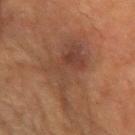Captured during whole-body skin photography for melanoma surveillance; the lesion was not biopsied.
The lesion is on the right forearm.
A female patient, roughly 80 years of age.
A lesion tile, about 15 mm wide, cut from a 3D total-body photograph.